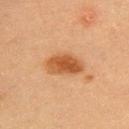Assessment: Recorded during total-body skin imaging; not selected for excision or biopsy. Image and clinical context: The tile uses cross-polarized illumination. A female subject, in their mid- to late 60s. A roughly 15 mm field-of-view crop from a total-body skin photograph. On the upper back. The recorded lesion diameter is about 5 mm.